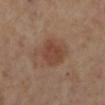The lesion was tiled from a total-body skin photograph and was not biopsied.